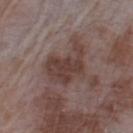The lesion was photographed on a routine skin check and not biopsied; there is no pathology result. Imaged with white-light lighting. Measured at roughly 5.5 mm in maximum diameter. A 15 mm close-up extracted from a 3D total-body photography capture. The patient is a male in their mid-60s. Located on the left upper arm. The total-body-photography lesion software estimated a mean CIELAB color near L≈39 a*≈17 b*≈21, roughly 10 lightness units darker than nearby skin, and a lesion-to-skin contrast of about 8.5 (normalized; higher = more distinct). And it measured an automated nevus-likeness rating near 0 out of 100 and lesion-presence confidence of about 100/100.